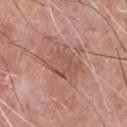* patient · male, aged around 60
* location · the chest
* automated lesion analysis · an area of roughly 12 mm², an eccentricity of roughly 0.65, and two-axis asymmetry of about 0.35; a lesion-detection confidence of about 95/100
* illumination · white-light
* imaging modality · 15 mm crop, total-body photography
* size · about 5 mm